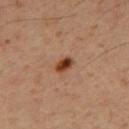{
  "biopsy_status": "not biopsied; imaged during a skin examination",
  "patient": {
    "sex": "male",
    "age_approx": 50
  },
  "image": {
    "source": "total-body photography crop",
    "field_of_view_mm": 15
  },
  "site": "back"
}